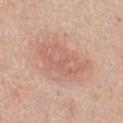Imaged during a routine full-body skin examination; the lesion was not biopsied and no histopathology is available. A close-up tile cropped from a whole-body skin photograph, about 15 mm across. Automated tile analysis of the lesion measured a mean CIELAB color near L≈63 a*≈21 b*≈27, about 7 CIELAB-L* units darker than the surrounding skin, and a normalized lesion–skin contrast near 5. And it measured lesion-presence confidence of about 100/100. Measured at roughly 8.5 mm in maximum diameter. On the front of the torso. A male patient, aged 43–47.